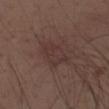  biopsy_status: not biopsied; imaged during a skin examination
  lighting: white-light
  lesion_size:
    long_diameter_mm_approx: 4.5
  image:
    source: total-body photography crop
    field_of_view_mm: 15
  site: back
  automated_metrics:
    cielab_L: 34
    cielab_a: 16
    cielab_b: 19
    vs_skin_darker_L: 5.0
    vs_skin_contrast_norm: 5.0
    border_irregularity_0_10: 3.0
    color_variation_0_10: 2.0
    peripheral_color_asymmetry: 0.5
  patient:
    sex: male
    age_approx: 30